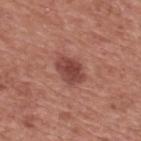Assessment:
Captured during whole-body skin photography for melanoma surveillance; the lesion was not biopsied.
Clinical summary:
Approximately 4 mm at its widest. An algorithmic analysis of the crop reported a footprint of about 7.5 mm², an outline eccentricity of about 0.75 (0 = round, 1 = elongated), and a symmetry-axis asymmetry near 0.15. The software also gave a lesion color around L≈44 a*≈26 b*≈25 in CIELAB and a lesion-to-skin contrast of about 8.5 (normalized; higher = more distinct). It also reported an automated nevus-likeness rating near 75 out of 100 and a detector confidence of about 100 out of 100 that the crop contains a lesion. The patient is a male roughly 75 years of age. Imaged with white-light lighting. The lesion is located on the upper back. A 15 mm close-up tile from a total-body photography series done for melanoma screening.